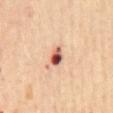Q: Was this lesion biopsied?
A: catalogued during a skin exam; not biopsied
Q: What is the imaging modality?
A: ~15 mm crop, total-body skin-cancer survey
Q: Patient demographics?
A: female, aged 63–67
Q: Where on the body is the lesion?
A: the mid back